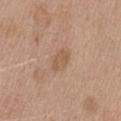Assessment: The lesion was photographed on a routine skin check and not biopsied; there is no pathology result. Context: The patient is a male aged 68–72. This image is a 15 mm lesion crop taken from a total-body photograph. The recorded lesion diameter is about 3 mm. From the back. Captured under white-light illumination. An algorithmic analysis of the crop reported a lesion color around L≈56 a*≈18 b*≈31 in CIELAB, a lesion–skin lightness drop of about 7, and a normalized lesion–skin contrast near 5.5. And it measured a nevus-likeness score of about 15/100.